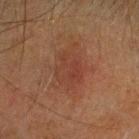Q: Was a biopsy performed?
A: no biopsy performed (imaged during a skin exam)
Q: What is the anatomic site?
A: the head or neck
Q: How was the tile lit?
A: cross-polarized
Q: What is the lesion's diameter?
A: ~4.5 mm (longest diameter)
Q: Automated lesion metrics?
A: a lesion area of about 10 mm², an eccentricity of roughly 0.7, and two-axis asymmetry of about 0.25
Q: What is the imaging modality?
A: 15 mm crop, total-body photography
Q: Patient demographics?
A: male, roughly 50 years of age